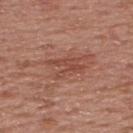– biopsy status · catalogued during a skin exam; not biopsied
– image source · ~15 mm tile from a whole-body skin photo
– subject · male, roughly 55 years of age
– anatomic site · the upper back